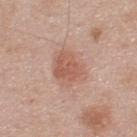The lesion was tiled from a total-body skin photograph and was not biopsied. This is a white-light tile. On the upper back. Automated image analysis of the tile measured a classifier nevus-likeness of about 85/100 and a detector confidence of about 100 out of 100 that the crop contains a lesion. A 15 mm crop from a total-body photograph taken for skin-cancer surveillance. About 4.5 mm across. A male patient, aged 43–47.